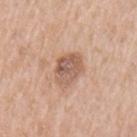A male subject, approximately 60 years of age. A 15 mm close-up extracted from a 3D total-body photography capture. Measured at roughly 4 mm in maximum diameter. The lesion is on the left upper arm.